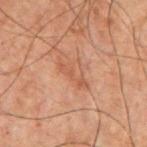Case summary:
- workup: catalogued during a skin exam; not biopsied
- subject: male, aged 68–72
- imaging modality: total-body-photography crop, ~15 mm field of view
- body site: the chest
- lesion diameter: about 4 mm